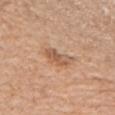Recorded during total-body skin imaging; not selected for excision or biopsy.
This is a white-light tile.
A female subject, in their 70s.
Located on the left upper arm.
Measured at roughly 3.5 mm in maximum diameter.
A 15 mm crop from a total-body photograph taken for skin-cancer surveillance.